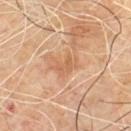<case>
<biopsy_status>not biopsied; imaged during a skin examination</biopsy_status>
<patient>
  <sex>male</sex>
  <age_approx>60</age_approx>
</patient>
<lighting>cross-polarized</lighting>
<lesion_size>
  <long_diameter_mm_approx>3.0</long_diameter_mm_approx>
</lesion_size>
<image>
  <source>total-body photography crop</source>
  <field_of_view_mm>15</field_of_view_mm>
</image>
<automated_metrics>
  <area_mm2_approx>4.5</area_mm2_approx>
  <eccentricity>0.8</eccentricity>
  <shape_asymmetry>0.45</shape_asymmetry>
  <nevus_likeness_0_100>0</nevus_likeness_0_100>
  <lesion_detection_confidence_0_100>100</lesion_detection_confidence_0_100>
</automated_metrics>
<site>upper back</site>
</case>